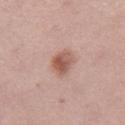Recorded during total-body skin imaging; not selected for excision or biopsy.